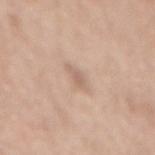• image — ~15 mm tile from a whole-body skin photo
• lesion diameter — ≈3 mm
• site — the mid back
• tile lighting — white-light
• subject — female, roughly 60 years of age